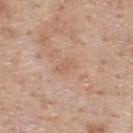follow-up: total-body-photography surveillance lesion; no biopsy
location: the upper back
patient: male, approximately 60 years of age
image source: ~15 mm crop, total-body skin-cancer survey
lighting: white-light illumination